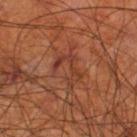biopsy status=imaged on a skin check; not biopsied
automated lesion analysis=a lesion color around L≈37 a*≈25 b*≈30 in CIELAB and a normalized border contrast of about 6; an automated nevus-likeness rating near 0 out of 100 and a lesion-detection confidence of about 85/100
location=the right thigh
image=total-body-photography crop, ~15 mm field of view
patient=male, in their 70s
lesion diameter=≈3.5 mm